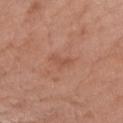biopsy_status: not biopsied; imaged during a skin examination
site: arm
patient:
  sex: female
  age_approx: 70
image:
  source: total-body photography crop
  field_of_view_mm: 15